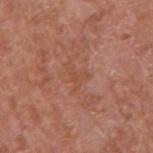This lesion was catalogued during total-body skin photography and was not selected for biopsy. A close-up tile cropped from a whole-body skin photograph, about 15 mm across. A male subject roughly 65 years of age. The lesion is located on the arm. Automated tile analysis of the lesion measured an area of roughly 3.5 mm², a shape eccentricity near 0.8, and two-axis asymmetry of about 0.7. The analysis additionally found a color-variation rating of about 0.5/10.